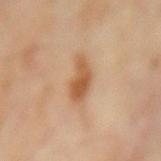| field | value |
|---|---|
| image source | total-body-photography crop, ~15 mm field of view |
| subject | male, aged around 65 |
| location | the mid back |
| lesion diameter | about 4.5 mm |